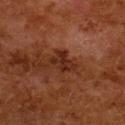Part of a total-body skin-imaging series; this lesion was reviewed on a skin check and was not flagged for biopsy. The lesion is located on the upper back. A 15 mm crop from a total-body photograph taken for skin-cancer surveillance. A female patient, approximately 50 years of age.